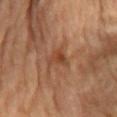• workup — catalogued during a skin exam; not biopsied
• lesion diameter — about 2.5 mm
• patient — male, aged 83 or older
• site — the mid back
• acquisition — 15 mm crop, total-body photography